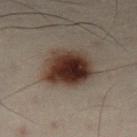Assessment:
Recorded during total-body skin imaging; not selected for excision or biopsy.
Image and clinical context:
This image is a 15 mm lesion crop taken from a total-body photograph. The lesion is located on the left lower leg. A male patient aged 48–52.